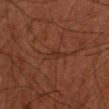{"biopsy_status": "not biopsied; imaged during a skin examination", "lesion_size": {"long_diameter_mm_approx": 2.5}, "site": "right forearm", "patient": {"sex": "male", "age_approx": 70}, "image": {"source": "total-body photography crop", "field_of_view_mm": 15}, "lighting": "cross-polarized"}